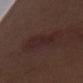Notes:
* biopsy status: no biopsy performed (imaged during a skin exam)
* automated metrics: an area of roughly 8 mm² and two-axis asymmetry of about 0.2; a lesion color around L≈23 a*≈17 b*≈17 in CIELAB and roughly 5 lightness units darker than nearby skin; a peripheral color-asymmetry measure near 0.5
* lesion diameter: ≈5 mm
* site: the left upper arm
* lighting: white-light
* subject: male, aged 68–72
* imaging modality: 15 mm crop, total-body photography